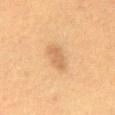{"biopsy_status": "not biopsied; imaged during a skin examination", "patient": {"sex": "female", "age_approx": 55}, "image": {"source": "total-body photography crop", "field_of_view_mm": 15}, "lesion_size": {"long_diameter_mm_approx": 3.0}, "site": "abdomen", "automated_metrics": {"area_mm2_approx": 4.0, "shape_asymmetry": 0.25}, "lighting": "cross-polarized"}